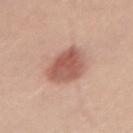lesion size = ~5.5 mm (longest diameter); site = the leg; imaging modality = ~15 mm crop, total-body skin-cancer survey; patient = male, aged approximately 60.